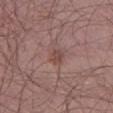Q: Was this lesion biopsied?
A: total-body-photography surveillance lesion; no biopsy